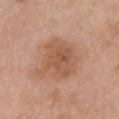automated metrics: a lesion area of about 18 mm², a shape eccentricity near 0.55, and two-axis asymmetry of about 0.25; an average lesion color of about L≈55 a*≈22 b*≈32 (CIELAB), roughly 9 lightness units darker than nearby skin, and a lesion-to-skin contrast of about 6.5 (normalized; higher = more distinct); an automated nevus-likeness rating near 10 out of 100 and lesion-presence confidence of about 100/100
subject: female, aged around 65
size: ~5.5 mm (longest diameter)
acquisition: ~15 mm crop, total-body skin-cancer survey
body site: the chest